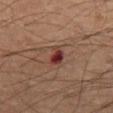Part of a total-body skin-imaging series; this lesion was reviewed on a skin check and was not flagged for biopsy. Approximately 3 mm at its widest. A 15 mm close-up extracted from a 3D total-body photography capture. A male patient aged approximately 65. The lesion is located on the mid back. The tile uses cross-polarized illumination.